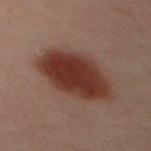Part of a total-body skin-imaging series; this lesion was reviewed on a skin check and was not flagged for biopsy. Measured at roughly 8 mm in maximum diameter. On the chest. A roughly 15 mm field-of-view crop from a total-body skin photograph. The tile uses cross-polarized illumination. The total-body-photography lesion software estimated an area of roughly 26 mm² and a shape-asymmetry score of about 0.1 (0 = symmetric). The patient is a male in their 40s.